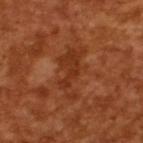  biopsy_status: not biopsied; imaged during a skin examination
  automated_metrics:
    area_mm2_approx: 12.0
    shape_asymmetry: 0.45
    cielab_L: 34
    cielab_a: 26
    cielab_b: 34
    vs_skin_darker_L: 6.0
    vs_skin_contrast_norm: 6.0
    border_irregularity_0_10: 6.5
    peripheral_color_asymmetry: 0.5
    nevus_likeness_0_100: 0
    lesion_detection_confidence_0_100: 90
  lesion_size:
    long_diameter_mm_approx: 6.0
  patient:
    sex: male
    age_approx: 65
  image:
    source: total-body photography crop
    field_of_view_mm: 15
  lighting: cross-polarized